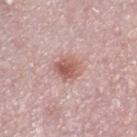Assessment:
Captured during whole-body skin photography for melanoma surveillance; the lesion was not biopsied.
Acquisition and patient details:
Approximately 3.5 mm at its widest. A 15 mm close-up extracted from a 3D total-body photography capture. Imaged with white-light lighting. On the right lower leg. The patient is a female approximately 40 years of age.